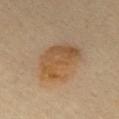Q: Is there a histopathology result?
A: catalogued during a skin exam; not biopsied